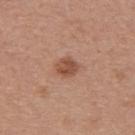body site — the upper back | automated metrics — border irregularity of about 1 on a 0–10 scale and radial color variation of about 1; lesion-presence confidence of about 100/100 | patient — female, aged approximately 35 | illumination — white-light illumination | lesion diameter — ~2.5 mm (longest diameter) | imaging modality — ~15 mm tile from a whole-body skin photo.